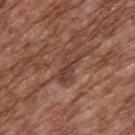Q: Was a biopsy performed?
A: no biopsy performed (imaged during a skin exam)
Q: How was this image acquired?
A: total-body-photography crop, ~15 mm field of view
Q: What is the lesion's diameter?
A: ~4 mm (longest diameter)
Q: What are the patient's age and sex?
A: male, in their mid- to late 70s
Q: How was the tile lit?
A: white-light illumination
Q: Lesion location?
A: the upper back